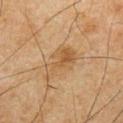The lesion was tiled from a total-body skin photograph and was not biopsied.
A male patient roughly 65 years of age.
The lesion is on the front of the torso.
Automated tile analysis of the lesion measured a mean CIELAB color near L≈47 a*≈16 b*≈34 and a normalized lesion–skin contrast near 6. The software also gave border irregularity of about 6.5 on a 0–10 scale.
Captured under cross-polarized illumination.
A 15 mm crop from a total-body photograph taken for skin-cancer surveillance.
Approximately 5 mm at its widest.